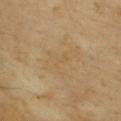Impression: The lesion was tiled from a total-body skin photograph and was not biopsied. Background: A region of skin cropped from a whole-body photographic capture, roughly 15 mm wide. The lesion-visualizer software estimated an eccentricity of roughly 0.8 and a symmetry-axis asymmetry near 0.5. And it measured a within-lesion color-variation index near 2.5/10 and radial color variation of about 0.5. From the chest. The patient is a male aged 63 to 67.